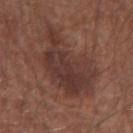Assessment:
The lesion was tiled from a total-body skin photograph and was not biopsied.
Background:
The lesion is on the left forearm. The lesion-visualizer software estimated a footprint of about 33 mm², an eccentricity of roughly 0.75, and a symmetry-axis asymmetry near 0.35. The software also gave a border-irregularity rating of about 5.5/10, a color-variation rating of about 4/10, and radial color variation of about 1.5. It also reported a classifier nevus-likeness of about 45/100. A male subject, about 65 years old. A roughly 15 mm field-of-view crop from a total-body skin photograph.